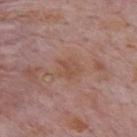Case summary:
- workup: imaged on a skin check; not biopsied
- tile lighting: white-light illumination
- image source: 15 mm crop, total-body photography
- anatomic site: the mid back
- size: ~2.5 mm (longest diameter)
- patient: male, roughly 75 years of age